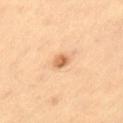This lesion was catalogued during total-body skin photography and was not selected for biopsy.
From the left thigh.
About 2 mm across.
A roughly 15 mm field-of-view crop from a total-body skin photograph.
A male patient, about 55 years old.